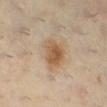This lesion was catalogued during total-body skin photography and was not selected for biopsy. The subject is a female in their mid-40s. A close-up tile cropped from a whole-body skin photograph, about 15 mm across. The lesion is on the right lower leg. Measured at roughly 4.5 mm in maximum diameter. The tile uses cross-polarized illumination.